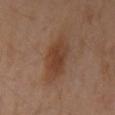Clinical impression:
Captured during whole-body skin photography for melanoma surveillance; the lesion was not biopsied.
Image and clinical context:
Approximately 5 mm at its widest. The subject is a female aged 43–47. Located on the left upper arm. A 15 mm close-up tile from a total-body photography series done for melanoma screening. The tile uses cross-polarized illumination.